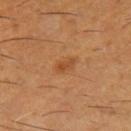Impression:
The lesion was tiled from a total-body skin photograph and was not biopsied.
Acquisition and patient details:
About 2 mm across. On the leg. A male subject about 60 years old. The total-body-photography lesion software estimated a footprint of about 2 mm², a shape eccentricity near 0.9, and two-axis asymmetry of about 0.3. It also reported a lesion color around L≈44 a*≈24 b*≈38 in CIELAB and roughly 8 lightness units darker than nearby skin. The software also gave a border-irregularity rating of about 3/10 and peripheral color asymmetry of about 0. And it measured a nevus-likeness score of about 70/100 and a lesion-detection confidence of about 100/100. Cropped from a total-body skin-imaging series; the visible field is about 15 mm.